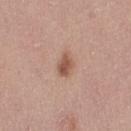  biopsy_status: not biopsied; imaged during a skin examination
  image:
    source: total-body photography crop
    field_of_view_mm: 15
  lesion_size:
    long_diameter_mm_approx: 2.5
  patient:
    sex: female
    age_approx: 45
  lighting: white-light
  automated_metrics:
    area_mm2_approx: 4.5
    eccentricity: 0.7
    shape_asymmetry: 0.2
    cielab_L: 54
    cielab_a: 21
    cielab_b: 29
    vs_skin_darker_L: 11.0
    vs_skin_contrast_norm: 8.0
    peripheral_color_asymmetry: 1.0
    nevus_likeness_0_100: 95
    lesion_detection_confidence_0_100: 100
  site: right thigh